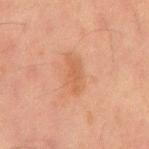<lesion>
  <biopsy_status>not biopsied; imaged during a skin examination</biopsy_status>
  <lighting>cross-polarized</lighting>
  <automated_metrics>
    <area_mm2_approx>6.0</area_mm2_approx>
    <eccentricity>0.95</eccentricity>
    <shape_asymmetry>0.3</shape_asymmetry>
    <cielab_L>47</cielab_L>
    <cielab_a>21</cielab_a>
    <cielab_b>30</cielab_b>
    <vs_skin_darker_L>6.0</vs_skin_darker_L>
    <vs_skin_contrast_norm>5.5</vs_skin_contrast_norm>
    <border_irregularity_0_10>4.5</border_irregularity_0_10>
    <peripheral_color_asymmetry>0.5</peripheral_color_asymmetry>
  </automated_metrics>
  <patient>
    <sex>male</sex>
    <age_approx>65</age_approx>
  </patient>
  <image>
    <source>total-body photography crop</source>
    <field_of_view_mm>15</field_of_view_mm>
  </image>
  <lesion_size>
    <long_diameter_mm_approx>4.5</long_diameter_mm_approx>
  </lesion_size>
  <site>abdomen</site>
</lesion>